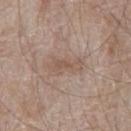Imaged during a routine full-body skin examination; the lesion was not biopsied and no histopathology is available.
Approximately 4 mm at its widest.
From the chest.
Automated tile analysis of the lesion measured a lesion area of about 6 mm², an outline eccentricity of about 0.9 (0 = round, 1 = elongated), and two-axis asymmetry of about 0.35. The software also gave a lesion–skin lightness drop of about 7 and a lesion-to-skin contrast of about 5.5 (normalized; higher = more distinct). It also reported a border-irregularity rating of about 4.5/10 and a color-variation rating of about 2/10. And it measured an automated nevus-likeness rating near 0 out of 100 and a lesion-detection confidence of about 100/100.
A 15 mm crop from a total-body photograph taken for skin-cancer surveillance.
This is a white-light tile.
The patient is a male aged 63 to 67.